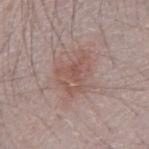follow-up: no biopsy performed (imaged during a skin exam)
lesion size: ≈4.5 mm
acquisition: 15 mm crop, total-body photography
patient: male, roughly 25 years of age
location: the right forearm
lighting: white-light illumination